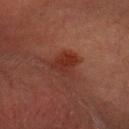* site · the head or neck
* image-analysis metrics · a lesion area of about 7 mm², an outline eccentricity of about 0.7 (0 = round, 1 = elongated), and a shape-asymmetry score of about 0.35 (0 = symmetric)
* subject · male, aged 48–52
* illumination · cross-polarized
* image · total-body-photography crop, ~15 mm field of view